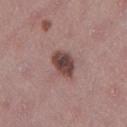No biopsy was performed on this lesion — it was imaged during a full skin examination and was not determined to be concerning.
This is a white-light tile.
Automated tile analysis of the lesion measured an area of roughly 7.5 mm², a shape eccentricity near 0.6, and a shape-asymmetry score of about 0.15 (0 = symmetric). The analysis additionally found a lesion color around L≈44 a*≈20 b*≈20 in CIELAB, roughly 14 lightness units darker than nearby skin, and a normalized border contrast of about 10.5. It also reported a border-irregularity rating of about 1.5/10, internal color variation of about 5.5 on a 0–10 scale, and radial color variation of about 1.5.
The lesion is on the leg.
About 3.5 mm across.
A close-up tile cropped from a whole-body skin photograph, about 15 mm across.
A female subject, in their mid- to late 40s.